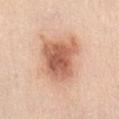biopsy status=total-body-photography surveillance lesion; no biopsy | image-analysis metrics=an area of roughly 21 mm²; an average lesion color of about L≈61 a*≈24 b*≈32 (CIELAB), about 15 CIELAB-L* units darker than the surrounding skin, and a normalized border contrast of about 9; an automated nevus-likeness rating near 95 out of 100 and a lesion-detection confidence of about 100/100 | subject=female, approximately 45 years of age | diameter=~6 mm (longest diameter) | image=~15 mm tile from a whole-body skin photo | anatomic site=the front of the torso.